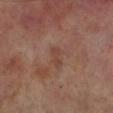Assessment: Part of a total-body skin-imaging series; this lesion was reviewed on a skin check and was not flagged for biopsy. Clinical summary: A roughly 15 mm field-of-view crop from a total-body skin photograph. An algorithmic analysis of the crop reported a lesion color around L≈41 a*≈20 b*≈26 in CIELAB, a lesion–skin lightness drop of about 6, and a normalized lesion–skin contrast near 5. The patient is a male roughly 70 years of age. From the leg.